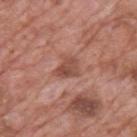– workup: catalogued during a skin exam; not biopsied
– patient: male, in their 70s
– image source: ~15 mm crop, total-body skin-cancer survey
– illumination: white-light illumination
– body site: the back